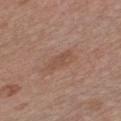Q: Is there a histopathology result?
A: total-body-photography surveillance lesion; no biopsy
Q: How was this image acquired?
A: total-body-photography crop, ~15 mm field of view
Q: Lesion location?
A: the front of the torso
Q: How was the tile lit?
A: white-light illumination
Q: Patient demographics?
A: female, about 40 years old
Q: What did automated image analysis measure?
A: a footprint of about 4 mm² and an eccentricity of roughly 0.85; a lesion color around L≈50 a*≈19 b*≈28 in CIELAB, a lesion–skin lightness drop of about 6, and a normalized border contrast of about 5; a border-irregularity index near 2.5/10, internal color variation of about 1 on a 0–10 scale, and peripheral color asymmetry of about 0.5